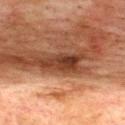Q: What is the imaging modality?
A: 15 mm crop, total-body photography
Q: How large is the lesion?
A: ~5 mm (longest diameter)
Q: Patient demographics?
A: male, roughly 75 years of age
Q: Lesion location?
A: the back